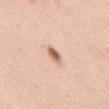{"biopsy_status": "not biopsied; imaged during a skin examination", "lighting": "white-light", "lesion_size": {"long_diameter_mm_approx": 2.5}, "automated_metrics": {"area_mm2_approx": 3.0, "eccentricity": 0.85, "shape_asymmetry": 0.2, "color_variation_0_10": 2.5, "peripheral_color_asymmetry": 1.0}, "site": "mid back", "image": {"source": "total-body photography crop", "field_of_view_mm": 15}, "patient": {"sex": "female", "age_approx": 50}}